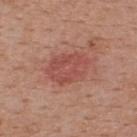| key | value |
|---|---|
| image-analysis metrics | an average lesion color of about L≈50 a*≈27 b*≈27 (CIELAB), about 8 CIELAB-L* units darker than the surrounding skin, and a lesion-to-skin contrast of about 6 (normalized; higher = more distinct); a border-irregularity rating of about 2/10 and a peripheral color-asymmetry measure near 1; a classifier nevus-likeness of about 70/100 and a lesion-detection confidence of about 100/100 |
| lesion diameter | about 5.5 mm |
| image source | 15 mm crop, total-body photography |
| lighting | white-light |
| body site | the back |
| patient | male, aged around 55 |